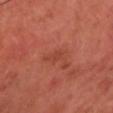This lesion was catalogued during total-body skin photography and was not selected for biopsy.
The tile uses cross-polarized illumination.
A male patient, approximately 70 years of age.
This image is a 15 mm lesion crop taken from a total-body photograph.
The lesion-visualizer software estimated a lesion area of about 1 mm², an eccentricity of roughly 0.55, and a shape-asymmetry score of about 0.3 (0 = symmetric). The software also gave a lesion color around L≈41 a*≈30 b*≈32 in CIELAB, roughly 5 lightness units darker than nearby skin, and a lesion-to-skin contrast of about 4 (normalized; higher = more distinct). And it measured a border-irregularity index near 2/10 and peripheral color asymmetry of about 0.
Longest diameter approximately 1 mm.
On the head or neck.